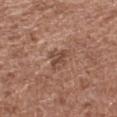Findings:
– workup · catalogued during a skin exam; not biopsied
– subject · female, approximately 75 years of age
– anatomic site · the left thigh
– automated lesion analysis · an area of roughly 4.5 mm² and an outline eccentricity of about 0.8 (0 = round, 1 = elongated); a border-irregularity index near 5/10, a within-lesion color-variation index near 3/10, and radial color variation of about 1
– image source · ~15 mm crop, total-body skin-cancer survey